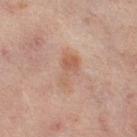biopsy status: imaged on a skin check; not biopsied
location: the left thigh
image source: total-body-photography crop, ~15 mm field of view
patient: female, in their 50s